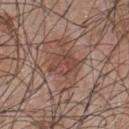Clinical impression: This lesion was catalogued during total-body skin photography and was not selected for biopsy. Background: This is a white-light tile. Measured at roughly 4 mm in maximum diameter. The subject is a male aged 43–47. This image is a 15 mm lesion crop taken from a total-body photograph. The lesion is located on the chest.